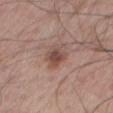Imaged with white-light lighting.
A lesion tile, about 15 mm wide, cut from a 3D total-body photograph.
Approximately 4 mm at its widest.
Automated tile analysis of the lesion measured a mean CIELAB color near L≈49 a*≈19 b*≈23, about 10 CIELAB-L* units darker than the surrounding skin, and a normalized lesion–skin contrast near 7. The software also gave an automated nevus-likeness rating near 80 out of 100 and a lesion-detection confidence of about 100/100.
The lesion is on the mid back.
The patient is a male aged around 70.